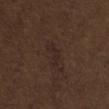biopsy_status: not biopsied; imaged during a skin examination
lesion_size:
  long_diameter_mm_approx: 3.5
image:
  source: total-body photography crop
  field_of_view_mm: 15
lighting: white-light
site: front of the torso
automated_metrics:
  eccentricity: 0.9
  shape_asymmetry: 0.5
  border_irregularity_0_10: 6.0
  color_variation_0_10: 0.0
  peripheral_color_asymmetry: 0.0
  nevus_likeness_0_100: 5
  lesion_detection_confidence_0_100: 85
patient:
  sex: male
  age_approx: 70